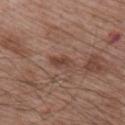notes = no biopsy performed (imaged during a skin exam)
lighting = white-light
image = ~15 mm crop, total-body skin-cancer survey
diameter = ~3 mm (longest diameter)
location = the right upper arm
patient = male, roughly 65 years of age